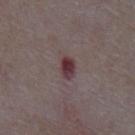Recorded during total-body skin imaging; not selected for excision or biopsy.
About 3 mm across.
The lesion is located on the abdomen.
Cropped from a whole-body photographic skin survey; the tile spans about 15 mm.
A male patient, aged 63–67.